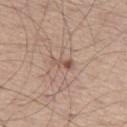Impression: Imaged during a routine full-body skin examination; the lesion was not biopsied and no histopathology is available. Context: About 2.5 mm across. A roughly 15 mm field-of-view crop from a total-body skin photograph. Located on the left thigh. A male subject, aged approximately 60.